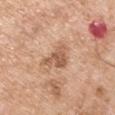This lesion was catalogued during total-body skin photography and was not selected for biopsy. Measured at roughly 3.5 mm in maximum diameter. A male patient, aged around 55. The lesion is on the left upper arm. Captured under white-light illumination. Automated image analysis of the tile measured an average lesion color of about L≈58 a*≈21 b*≈32 (CIELAB) and a lesion-to-skin contrast of about 7 (normalized; higher = more distinct). The analysis additionally found border irregularity of about 5 on a 0–10 scale and radial color variation of about 1.5. A lesion tile, about 15 mm wide, cut from a 3D total-body photograph.